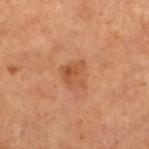This lesion was catalogued during total-body skin photography and was not selected for biopsy. Imaged with cross-polarized lighting. A female subject, aged around 55. From the left lower leg. Cropped from a whole-body photographic skin survey; the tile spans about 15 mm. Approximately 3 mm at its widest.